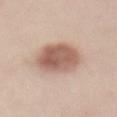– notes — catalogued during a skin exam; not biopsied
– image — total-body-photography crop, ~15 mm field of view
– size — about 5.5 mm
– anatomic site — the back
– patient — female, in their 50s
– lighting — white-light